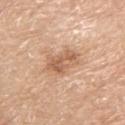Q: Was this lesion biopsied?
A: total-body-photography surveillance lesion; no biopsy
Q: Lesion location?
A: the right upper arm
Q: Who is the patient?
A: male, aged approximately 70
Q: What is the imaging modality?
A: ~15 mm crop, total-body skin-cancer survey